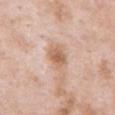notes=total-body-photography surveillance lesion; no biopsy | patient=male, in their mid-50s | anatomic site=the arm | image source=~15 mm tile from a whole-body skin photo.